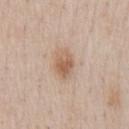  biopsy_status: not biopsied; imaged during a skin examination
  patient:
    sex: male
    age_approx: 60
  image:
    source: total-body photography crop
    field_of_view_mm: 15
  site: chest
  lesion_size:
    long_diameter_mm_approx: 3.5